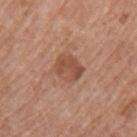Assessment: No biopsy was performed on this lesion — it was imaged during a full skin examination and was not determined to be concerning. Context: The patient is a female roughly 60 years of age. About 3.5 mm across. Located on the back. A close-up tile cropped from a whole-body skin photograph, about 15 mm across.